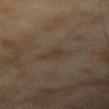Notes:
* workup — catalogued during a skin exam; not biopsied
* subject — male, aged 43 to 47
* TBP lesion metrics — a footprint of about 5 mm² and a shape-asymmetry score of about 0.3 (0 = symmetric); a border-irregularity rating of about 2.5/10, internal color variation of about 1.5 on a 0–10 scale, and radial color variation of about 0.5
* lighting — cross-polarized
* image source — total-body-photography crop, ~15 mm field of view
* lesion size — about 2.5 mm
* body site — the right forearm